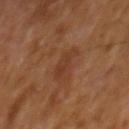notes: catalogued during a skin exam; not biopsied | subject: male, about 65 years old | diameter: ≈3.5 mm | lighting: cross-polarized | location: the mid back | automated lesion analysis: a footprint of about 4.5 mm² and a shape eccentricity near 0.9; border irregularity of about 4.5 on a 0–10 scale, internal color variation of about 1.5 on a 0–10 scale, and radial color variation of about 0.5 | imaging modality: total-body-photography crop, ~15 mm field of view.